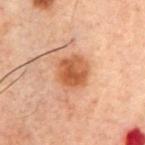This lesion was catalogued during total-body skin photography and was not selected for biopsy. A male subject, roughly 60 years of age. From the chest. Cropped from a total-body skin-imaging series; the visible field is about 15 mm.